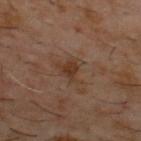{
  "biopsy_status": "not biopsied; imaged during a skin examination",
  "site": "upper back",
  "patient": {
    "sex": "male",
    "age_approx": 60
  },
  "automated_metrics": {
    "area_mm2_approx": 6.0,
    "eccentricity": 0.7,
    "shape_asymmetry": 0.55,
    "vs_skin_darker_L": 6.0,
    "vs_skin_contrast_norm": 7.0,
    "border_irregularity_0_10": 6.0,
    "color_variation_0_10": 2.0,
    "peripheral_color_asymmetry": 0.5,
    "nevus_likeness_0_100": 0,
    "lesion_detection_confidence_0_100": 100
  },
  "image": {
    "source": "total-body photography crop",
    "field_of_view_mm": 15
  },
  "lighting": "cross-polarized",
  "lesion_size": {
    "long_diameter_mm_approx": 3.5
  }
}